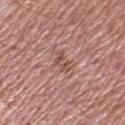Recorded during total-body skin imaging; not selected for excision or biopsy. Imaged with white-light lighting. A 15 mm crop from a total-body photograph taken for skin-cancer surveillance. The lesion is located on the arm. A male subject, aged 43 to 47. About 3 mm across.